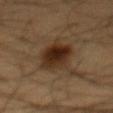Captured during whole-body skin photography for melanoma surveillance; the lesion was not biopsied. The tile uses cross-polarized illumination. A 15 mm crop from a total-body photograph taken for skin-cancer surveillance. An algorithmic analysis of the crop reported a footprint of about 16 mm², a shape eccentricity near 0.9, and a symmetry-axis asymmetry near 0.15. The analysis additionally found a mean CIELAB color near L≈27 a*≈15 b*≈25, about 11 CIELAB-L* units darker than the surrounding skin, and a lesion-to-skin contrast of about 11 (normalized; higher = more distinct). A male subject approximately 60 years of age. The lesion is on the abdomen. The recorded lesion diameter is about 7 mm.